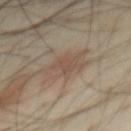<tbp_lesion>
<biopsy_status>not biopsied; imaged during a skin examination</biopsy_status>
<lesion_size>
  <long_diameter_mm_approx>2.0</long_diameter_mm_approx>
</lesion_size>
<patient>
  <sex>male</sex>
  <age_approx>40</age_approx>
</patient>
<image>
  <source>total-body photography crop</source>
  <field_of_view_mm>15</field_of_view_mm>
</image>
<site>mid back</site>
<automated_metrics>
  <cielab_L>49</cielab_L>
  <cielab_a>16</cielab_a>
  <cielab_b>26</cielab_b>
  <vs_skin_darker_L>5.0</vs_skin_darker_L>
  <vs_skin_contrast_norm>4.0</vs_skin_contrast_norm>
  <border_irregularity_0_10>2.0</border_irregularity_0_10>
  <color_variation_0_10>0.5</color_variation_0_10>
  <peripheral_color_asymmetry>0.0</peripheral_color_asymmetry>
</automated_metrics>
</tbp_lesion>